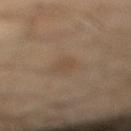• workup: imaged on a skin check; not biopsied
• image source: total-body-photography crop, ~15 mm field of view
• subject: male, aged 63–67
• lesion diameter: about 3 mm
• anatomic site: the right lower leg